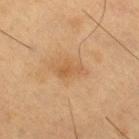Captured during whole-body skin photography for melanoma surveillance; the lesion was not biopsied.
A male subject, aged approximately 55.
A 15 mm close-up tile from a total-body photography series done for melanoma screening.
On the right thigh.
This is a cross-polarized tile.